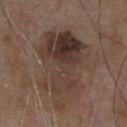{
  "biopsy_status": "not biopsied; imaged during a skin examination",
  "automated_metrics": {
    "border_irregularity_0_10": 5.0,
    "color_variation_0_10": 8.0
  },
  "site": "chest",
  "lighting": "white-light",
  "lesion_size": {
    "long_diameter_mm_approx": 9.5
  },
  "patient": {
    "sex": "male",
    "age_approx": 80
  },
  "image": {
    "source": "total-body photography crop",
    "field_of_view_mm": 15
  }
}